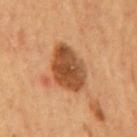Notes:
* subject: male, roughly 55 years of age
* lesion size: ≈6 mm
* tile lighting: cross-polarized
* image: 15 mm crop, total-body photography
* site: the mid back
* automated metrics: a lesion area of about 16 mm², an outline eccentricity of about 0.8 (0 = round, 1 = elongated), and two-axis asymmetry of about 0.15; a classifier nevus-likeness of about 55/100 and lesion-presence confidence of about 100/100